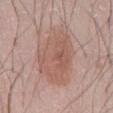<case>
<biopsy_status>not biopsied; imaged during a skin examination</biopsy_status>
<site>abdomen</site>
<lesion_size>
  <long_diameter_mm_approx>8.5</long_diameter_mm_approx>
</lesion_size>
<lighting>white-light</lighting>
<image>
  <source>total-body photography crop</source>
  <field_of_view_mm>15</field_of_view_mm>
</image>
<patient>
  <sex>male</sex>
  <age_approx>50</age_approx>
</patient>
</case>